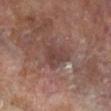Case summary:
– biopsy status · total-body-photography surveillance lesion; no biopsy
– acquisition · ~15 mm crop, total-body skin-cancer survey
– site · the left lower leg
– subject · female, in their mid- to late 70s
– illumination · cross-polarized illumination
– image-analysis metrics · a lesion area of about 8 mm², an outline eccentricity of about 0.55 (0 = round, 1 = elongated), and a symmetry-axis asymmetry near 0.35; about 8 CIELAB-L* units darker than the surrounding skin; border irregularity of about 3.5 on a 0–10 scale, a color-variation rating of about 3/10, and a peripheral color-asymmetry measure near 1; an automated nevus-likeness rating near 0 out of 100
– lesion size · ≈4 mm